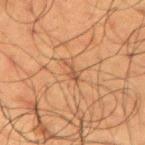Captured during whole-body skin photography for melanoma surveillance; the lesion was not biopsied. About 3 mm across. A male subject, approximately 60 years of age. Captured under cross-polarized illumination. A roughly 15 mm field-of-view crop from a total-body skin photograph. The lesion is located on the left upper arm.